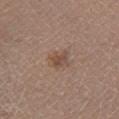follow-up = imaged on a skin check; not biopsied
location = the left lower leg
image = ~15 mm tile from a whole-body skin photo
subject = female, aged approximately 40